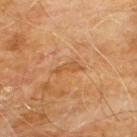Findings:
– patient · male, roughly 60 years of age
– tile lighting · cross-polarized
– lesion diameter · ≈3.5 mm
– image · 15 mm crop, total-body photography
– site · the chest